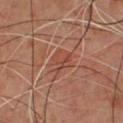Part of a total-body skin-imaging series; this lesion was reviewed on a skin check and was not flagged for biopsy. A male patient about 60 years old. Automated tile analysis of the lesion measured an average lesion color of about L≈44 a*≈25 b*≈28 (CIELAB). The software also gave border irregularity of about 4.5 on a 0–10 scale, a within-lesion color-variation index near 4.5/10, and radial color variation of about 1.5. It also reported a classifier nevus-likeness of about 0/100 and a lesion-detection confidence of about 80/100. On the front of the torso. A roughly 15 mm field-of-view crop from a total-body skin photograph. The tile uses cross-polarized illumination.